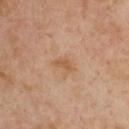This lesion was catalogued during total-body skin photography and was not selected for biopsy.